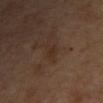Clinical impression:
The lesion was tiled from a total-body skin photograph and was not biopsied.
Context:
The lesion is on the right upper arm. This is a cross-polarized tile. The patient is about 60 years old. A lesion tile, about 15 mm wide, cut from a 3D total-body photograph. An algorithmic analysis of the crop reported a lesion area of about 3.5 mm², an outline eccentricity of about 0.9 (0 = round, 1 = elongated), and a shape-asymmetry score of about 0.4 (0 = symmetric). And it measured an average lesion color of about L≈28 a*≈15 b*≈24 (CIELAB) and roughly 4 lightness units darker than nearby skin. And it measured a border-irregularity index near 4/10, a color-variation rating of about 1/10, and a peripheral color-asymmetry measure near 0.5.